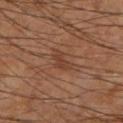{"biopsy_status": "not biopsied; imaged during a skin examination", "site": "leg", "image": {"source": "total-body photography crop", "field_of_view_mm": 15}, "lesion_size": {"long_diameter_mm_approx": 2.5}, "automated_metrics": {"area_mm2_approx": 4.0, "eccentricity": 0.75, "shape_asymmetry": 0.4, "cielab_L": 39, "cielab_a": 21, "cielab_b": 29, "vs_skin_darker_L": 7.0, "vs_skin_contrast_norm": 6.0, "border_irregularity_0_10": 4.0, "color_variation_0_10": 2.0, "peripheral_color_asymmetry": 0.5, "nevus_likeness_0_100": 0, "lesion_detection_confidence_0_100": 100}, "lighting": "cross-polarized", "patient": {"sex": "male", "age_approx": 60}}